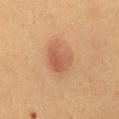Case summary:
– workup · total-body-photography surveillance lesion; no biopsy
– image · total-body-photography crop, ~15 mm field of view
– illumination · cross-polarized illumination
– patient · female, aged around 50
– size · about 4 mm
– anatomic site · the abdomen